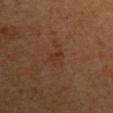Assessment:
The lesion was tiled from a total-body skin photograph and was not biopsied.
Acquisition and patient details:
Cropped from a whole-body photographic skin survey; the tile spans about 15 mm. The lesion is located on the arm. The subject is a male aged 58–62.